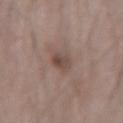This lesion was catalogued during total-body skin photography and was not selected for biopsy. About 3.5 mm across. The tile uses white-light illumination. A male patient, aged approximately 25. Cropped from a total-body skin-imaging series; the visible field is about 15 mm. On the left forearm.